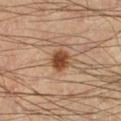Assessment: Captured during whole-body skin photography for melanoma surveillance; the lesion was not biopsied. Acquisition and patient details: A male patient aged 38 to 42. Captured under cross-polarized illumination. The lesion is located on the leg. Automated image analysis of the tile measured a within-lesion color-variation index near 3/10 and peripheral color asymmetry of about 1. The software also gave an automated nevus-likeness rating near 100 out of 100 and a lesion-detection confidence of about 100/100. Longest diameter approximately 3 mm. A 15 mm close-up extracted from a 3D total-body photography capture.